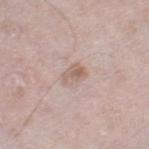Clinical impression: The lesion was photographed on a routine skin check and not biopsied; there is no pathology result. Clinical summary: The tile uses white-light illumination. Measured at roughly 3 mm in maximum diameter. The lesion is located on the right thigh. A lesion tile, about 15 mm wide, cut from a 3D total-body photograph. The subject is a male aged 63–67.